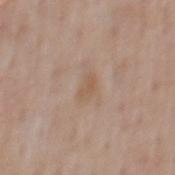Part of a total-body skin-imaging series; this lesion was reviewed on a skin check and was not flagged for biopsy. The total-body-photography lesion software estimated a border-irregularity index near 3/10, internal color variation of about 1.5 on a 0–10 scale, and a peripheral color-asymmetry measure near 0.5. Longest diameter approximately 3 mm. From the mid back. A roughly 15 mm field-of-view crop from a total-body skin photograph. Imaged with white-light lighting. The subject is a male about 55 years old.